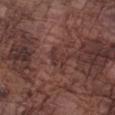<tbp_lesion>
  <biopsy_status>not biopsied; imaged during a skin examination</biopsy_status>
  <image>
    <source>total-body photography crop</source>
    <field_of_view_mm>15</field_of_view_mm>
  </image>
  <patient>
    <sex>male</sex>
    <age_approx>75</age_approx>
  </patient>
  <lesion_size>
    <long_diameter_mm_approx>2.5</long_diameter_mm_approx>
  </lesion_size>
  <site>arm</site>
</tbp_lesion>